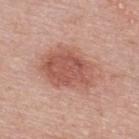<record>
  <biopsy_status>not biopsied; imaged during a skin examination</biopsy_status>
  <image>
    <source>total-body photography crop</source>
    <field_of_view_mm>15</field_of_view_mm>
  </image>
  <lesion_size>
    <long_diameter_mm_approx>7.5</long_diameter_mm_approx>
  </lesion_size>
  <lighting>white-light</lighting>
  <site>back</site>
  <patient>
    <sex>female</sex>
    <age_approx>50</age_approx>
  </patient>
  <automated_metrics>
    <cielab_L>56</cielab_L>
    <cielab_a>24</cielab_a>
    <cielab_b>28</cielab_b>
    <vs_skin_darker_L>11.0</vs_skin_darker_L>
    <vs_skin_contrast_norm>7.5</vs_skin_contrast_norm>
  </automated_metrics>
</record>